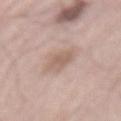Notes:
– follow-up: catalogued during a skin exam; not biopsied
– site: the mid back
– imaging modality: 15 mm crop, total-body photography
– patient: male, roughly 65 years of age